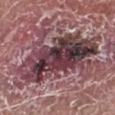follow-up: no biopsy performed (imaged during a skin exam) | image: ~15 mm tile from a whole-body skin photo | TBP lesion metrics: an area of roughly 42 mm², an eccentricity of roughly 0.9, and a shape-asymmetry score of about 0.25 (0 = symmetric); a lesion color around L≈38 a*≈21 b*≈13 in CIELAB, a lesion–skin lightness drop of about 16, and a lesion-to-skin contrast of about 13 (normalized; higher = more distinct) | patient: male, aged 78 to 82 | site: the left lower leg.